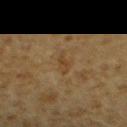The lesion was photographed on a routine skin check and not biopsied; there is no pathology result. A male patient, in their mid- to late 80s or older. Located on the chest. A roughly 15 mm field-of-view crop from a total-body skin photograph. Approximately 3 mm at its widest.